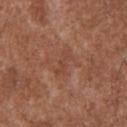<tbp_lesion>
<patient>
  <sex>male</sex>
  <age_approx>45</age_approx>
</patient>
<lighting>white-light</lighting>
<automated_metrics>
  <cielab_L>44</cielab_L>
  <cielab_a>23</cielab_a>
  <cielab_b>29</cielab_b>
  <vs_skin_darker_L>6.0</vs_skin_darker_L>
  <vs_skin_contrast_norm>4.5</vs_skin_contrast_norm>
  <nevus_likeness_0_100>0</nevus_likeness_0_100>
  <lesion_detection_confidence_0_100>100</lesion_detection_confidence_0_100>
</automated_metrics>
<image>
  <source>total-body photography crop</source>
  <field_of_view_mm>15</field_of_view_mm>
</image>
<site>upper back</site>
</tbp_lesion>